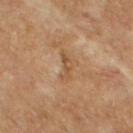Clinical impression: No biopsy was performed on this lesion — it was imaged during a full skin examination and was not determined to be concerning. Context: Located on the upper back. Automated image analysis of the tile measured internal color variation of about 3 on a 0–10 scale and a peripheral color-asymmetry measure near 1. A female subject in their 60s. Cropped from a whole-body photographic skin survey; the tile spans about 15 mm. The recorded lesion diameter is about 3.5 mm.